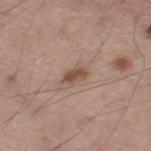workup: catalogued during a skin exam; not biopsied
location: the leg
acquisition: ~15 mm crop, total-body skin-cancer survey
subject: male, aged 53 to 57
size: about 3 mm
illumination: white-light illumination